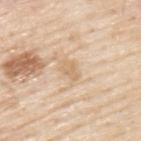No biopsy was performed on this lesion — it was imaged during a full skin examination and was not determined to be concerning.
Cropped from a total-body skin-imaging series; the visible field is about 15 mm.
Longest diameter approximately 2.5 mm.
Captured under white-light illumination.
A male patient, approximately 80 years of age.
On the upper back.